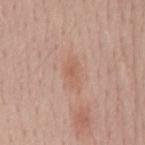Assessment:
This lesion was catalogued during total-body skin photography and was not selected for biopsy.
Background:
A male subject, aged 53 to 57. The lesion is located on the mid back. Cropped from a whole-body photographic skin survey; the tile spans about 15 mm. The lesion-visualizer software estimated a lesion color around L≈59 a*≈21 b*≈29 in CIELAB, roughly 6 lightness units darker than nearby skin, and a normalized border contrast of about 5. The analysis additionally found a within-lesion color-variation index near 2/10 and a peripheral color-asymmetry measure near 0.5. The analysis additionally found a nevus-likeness score of about 0/100 and a detector confidence of about 100 out of 100 that the crop contains a lesion.